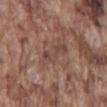| feature | finding |
|---|---|
| biopsy status | catalogued during a skin exam; not biopsied |
| image source | 15 mm crop, total-body photography |
| patient | male, in their mid- to late 70s |
| lighting | white-light illumination |
| lesion diameter | about 4.5 mm |
| site | the mid back |